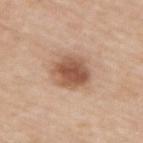workup = total-body-photography surveillance lesion; no biopsy | image-analysis metrics = a footprint of about 13 mm² and an eccentricity of roughly 0.65 | subject = male, aged 63 to 67 | lesion diameter = ~5 mm (longest diameter) | anatomic site = the back | imaging modality = ~15 mm crop, total-body skin-cancer survey.